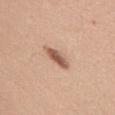Impression:
Recorded during total-body skin imaging; not selected for excision or biopsy.
Background:
A male subject, in their mid-50s. Approximately 4 mm at its widest. Automated image analysis of the tile measured a footprint of about 5 mm², a shape eccentricity near 0.9, and a shape-asymmetry score of about 0.25 (0 = symmetric). The analysis additionally found a border-irregularity index near 3/10, a within-lesion color-variation index near 3.5/10, and peripheral color asymmetry of about 1. Imaged with white-light lighting. Located on the upper back. A 15 mm close-up extracted from a 3D total-body photography capture.